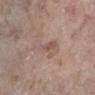Imaged during a routine full-body skin examination; the lesion was not biopsied and no histopathology is available.
The recorded lesion diameter is about 4.5 mm.
The lesion is located on the left lower leg.
The subject is a female aged 73–77.
This image is a 15 mm lesion crop taken from a total-body photograph.
Captured under white-light illumination.